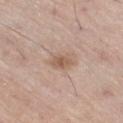Notes:
- notes · catalogued during a skin exam; not biopsied
- subject · male, roughly 70 years of age
- image · 15 mm crop, total-body photography
- body site · the right thigh
- lighting · white-light illumination
- lesion diameter · ~3 mm (longest diameter)
- image-analysis metrics · an area of roughly 4.5 mm² and an eccentricity of roughly 0.75; an average lesion color of about L≈57 a*≈17 b*≈28 (CIELAB) and about 9 CIELAB-L* units darker than the surrounding skin; border irregularity of about 2.5 on a 0–10 scale, a within-lesion color-variation index near 2.5/10, and a peripheral color-asymmetry measure near 0.5